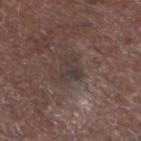Assessment:
No biopsy was performed on this lesion — it was imaged during a full skin examination and was not determined to be concerning.
Context:
The lesion is located on the left lower leg. Measured at roughly 3 mm in maximum diameter. A close-up tile cropped from a whole-body skin photograph, about 15 mm across. The tile uses white-light illumination. Automated tile analysis of the lesion measured an area of roughly 5 mm² and two-axis asymmetry of about 0.35. The software also gave border irregularity of about 4 on a 0–10 scale, a within-lesion color-variation index near 2.5/10, and a peripheral color-asymmetry measure near 1. A male patient, in their mid- to late 70s.